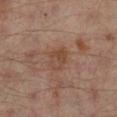The lesion was photographed on a routine skin check and not biopsied; there is no pathology result.
The patient is a male in their mid-60s.
A lesion tile, about 15 mm wide, cut from a 3D total-body photograph.
The recorded lesion diameter is about 3 mm.
This is a cross-polarized tile.
An algorithmic analysis of the crop reported a footprint of about 6 mm², an outline eccentricity of about 0.6 (0 = round, 1 = elongated), and a symmetry-axis asymmetry near 0.25. It also reported an average lesion color of about L≈43 a*≈18 b*≈27 (CIELAB) and a normalized border contrast of about 5.5.
From the right lower leg.